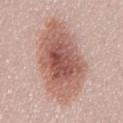notes: imaged on a skin check; not biopsied
lesion diameter: ≈11 mm
image: ~15 mm crop, total-body skin-cancer survey
subject: female, approximately 50 years of age
body site: the chest
image-analysis metrics: an eccentricity of roughly 0.9 and two-axis asymmetry of about 0.15; an average lesion color of about L≈58 a*≈22 b*≈25 (CIELAB) and a lesion-to-skin contrast of about 9 (normalized; higher = more distinct); a lesion-detection confidence of about 100/100